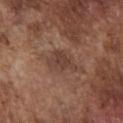{"biopsy_status": "not biopsied; imaged during a skin examination", "patient": {"sex": "male", "age_approx": 75}, "image": {"source": "total-body photography crop", "field_of_view_mm": 15}, "site": "chest"}